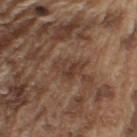Clinical impression:
Captured during whole-body skin photography for melanoma surveillance; the lesion was not biopsied.
Image and clinical context:
On the left upper arm. The patient is a male roughly 75 years of age. Measured at roughly 3.5 mm in maximum diameter. Automated image analysis of the tile measured an average lesion color of about L≈38 a*≈18 b*≈26 (CIELAB), a lesion–skin lightness drop of about 7, and a normalized border contrast of about 6.5. It also reported a border-irregularity rating of about 4.5/10 and internal color variation of about 4.5 on a 0–10 scale. The tile uses white-light illumination. A roughly 15 mm field-of-view crop from a total-body skin photograph.